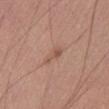Q: Is there a histopathology result?
A: total-body-photography surveillance lesion; no biopsy
Q: How large is the lesion?
A: ≈3 mm
Q: What is the anatomic site?
A: the abdomen
Q: What kind of image is this?
A: ~15 mm tile from a whole-body skin photo
Q: Patient demographics?
A: male, about 40 years old
Q: Illumination type?
A: white-light illumination